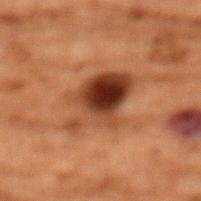<case>
  <biopsy_status>not biopsied; imaged during a skin examination</biopsy_status>
  <site>chest</site>
  <lesion_size>
    <long_diameter_mm_approx>6.0</long_diameter_mm_approx>
  </lesion_size>
  <patient>
    <sex>female</sex>
    <age_approx>80</age_approx>
  </patient>
  <lighting>cross-polarized</lighting>
  <image>
    <source>total-body photography crop</source>
    <field_of_view_mm>15</field_of_view_mm>
  </image>
</case>